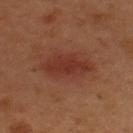Findings:
* follow-up — no biopsy performed (imaged during a skin exam)
* site — the upper back
* patient — male, aged around 50
* acquisition — total-body-photography crop, ~15 mm field of view
* diameter — ~5.5 mm (longest diameter)
* automated metrics — a lesion area of about 12 mm²; a mean CIELAB color near L≈34 a*≈26 b*≈28, roughly 8 lightness units darker than nearby skin, and a lesion-to-skin contrast of about 7 (normalized; higher = more distinct); a border-irregularity index near 2.5/10, a within-lesion color-variation index near 2.5/10, and a peripheral color-asymmetry measure near 1; an automated nevus-likeness rating near 95 out of 100 and a detector confidence of about 100 out of 100 that the crop contains a lesion